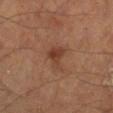The lesion was tiled from a total-body skin photograph and was not biopsied. A male patient about 65 years old. The lesion is located on the left lower leg. The recorded lesion diameter is about 3 mm. Imaged with cross-polarized lighting. A 15 mm close-up tile from a total-body photography series done for melanoma screening.